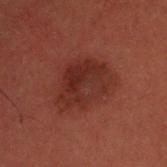Assessment:
Recorded during total-body skin imaging; not selected for excision or biopsy.
Context:
Imaged with cross-polarized lighting. Located on the head or neck. A 15 mm close-up extracted from a 3D total-body photography capture. A male patient, about 50 years old. The recorded lesion diameter is about 6 mm. The lesion-visualizer software estimated border irregularity of about 2.5 on a 0–10 scale, a within-lesion color-variation index near 4/10, and a peripheral color-asymmetry measure near 1.5. The analysis additionally found a nevus-likeness score of about 20/100.